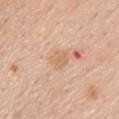| key | value |
|---|---|
| workup | imaged on a skin check; not biopsied |
| imaging modality | total-body-photography crop, ~15 mm field of view |
| tile lighting | white-light |
| patient | male, about 60 years old |
| anatomic site | the upper back |
| diameter | ~2.5 mm (longest diameter) |
| TBP lesion metrics | a footprint of about 4 mm², an outline eccentricity of about 0.55 (0 = round, 1 = elongated), and a shape-asymmetry score of about 0.25 (0 = symmetric); a border-irregularity rating of about 3/10 and radial color variation of about 0.5; a classifier nevus-likeness of about 0/100 and lesion-presence confidence of about 100/100 |